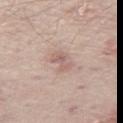Clinical impression: No biopsy was performed on this lesion — it was imaged during a full skin examination and was not determined to be concerning. Background: A 15 mm close-up extracted from a 3D total-body photography capture. The lesion is located on the left thigh. Imaged with white-light lighting. A male subject, in their mid- to late 60s.